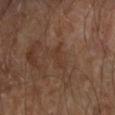{
  "biopsy_status": "not biopsied; imaged during a skin examination",
  "patient": {
    "sex": "male",
    "age_approx": "85+"
  },
  "lesion_size": {
    "long_diameter_mm_approx": 3.0
  },
  "lighting": "cross-polarized",
  "site": "right forearm",
  "image": {
    "source": "total-body photography crop",
    "field_of_view_mm": 15
  }
}